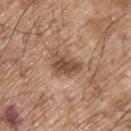No biopsy was performed on this lesion — it was imaged during a full skin examination and was not determined to be concerning.
A 15 mm close-up tile from a total-body photography series done for melanoma screening.
The recorded lesion diameter is about 4 mm.
Captured under white-light illumination.
On the upper back.
A male patient, roughly 65 years of age.